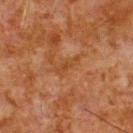Clinical impression: Imaged during a routine full-body skin examination; the lesion was not biopsied and no histopathology is available. Acquisition and patient details: A close-up tile cropped from a whole-body skin photograph, about 15 mm across. Automated image analysis of the tile measured a lesion area of about 3.5 mm², an eccentricity of roughly 0.95, and a shape-asymmetry score of about 0.4 (0 = symmetric). The analysis additionally found an average lesion color of about L≈35 a*≈20 b*≈31 (CIELAB), a lesion–skin lightness drop of about 5, and a lesion-to-skin contrast of about 5.5 (normalized; higher = more distinct). It also reported a border-irregularity rating of about 5/10, internal color variation of about 0 on a 0–10 scale, and a peripheral color-asymmetry measure near 0. The analysis additionally found a nevus-likeness score of about 0/100 and a detector confidence of about 85 out of 100 that the crop contains a lesion. A male subject aged 78 to 82. Approximately 3.5 mm at its widest. Located on the upper back.